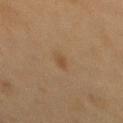Imaged during a routine full-body skin examination; the lesion was not biopsied and no histopathology is available. A 15 mm crop from a total-body photograph taken for skin-cancer surveillance. The subject is a female aged around 40. Approximately 2.5 mm at its widest. From the mid back. Imaged with cross-polarized lighting. An algorithmic analysis of the crop reported an area of roughly 3 mm², an outline eccentricity of about 0.85 (0 = round, 1 = elongated), and a symmetry-axis asymmetry near 0.2. And it measured a mean CIELAB color near L≈41 a*≈14 b*≈29. It also reported a border-irregularity rating of about 2/10, a color-variation rating of about 1/10, and a peripheral color-asymmetry measure near 0.5. And it measured a nevus-likeness score of about 5/100.